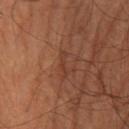biopsy_status: not biopsied; imaged during a skin examination
image:
  source: total-body photography crop
  field_of_view_mm: 15
site: left leg
patient:
  sex: male
  age_approx: 65